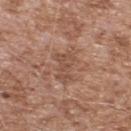Captured during whole-body skin photography for melanoma surveillance; the lesion was not biopsied. Automated image analysis of the tile measured a detector confidence of about 100 out of 100 that the crop contains a lesion. Approximately 4.5 mm at its widest. Located on the upper back. A male subject, aged around 55. A 15 mm crop from a total-body photograph taken for skin-cancer surveillance. Imaged with white-light lighting.